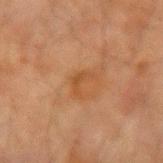{"biopsy_status": "not biopsied; imaged during a skin examination", "lesion_size": {"long_diameter_mm_approx": 3.0}, "image": {"source": "total-body photography crop", "field_of_view_mm": 15}, "patient": {"sex": "male", "age_approx": 65}, "site": "left forearm", "automated_metrics": {"cielab_L": 40, "cielab_a": 20, "cielab_b": 32, "vs_skin_darker_L": 5.0, "vs_skin_contrast_norm": 5.5}, "lighting": "cross-polarized"}